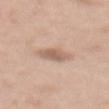Recorded during total-body skin imaging; not selected for excision or biopsy. A 15 mm close-up extracted from a 3D total-body photography capture. The lesion is located on the abdomen. The total-body-photography lesion software estimated an outline eccentricity of about 0.65 (0 = round, 1 = elongated) and a shape-asymmetry score of about 0.2 (0 = symmetric). The analysis additionally found an average lesion color of about L≈62 a*≈18 b*≈28 (CIELAB), about 10 CIELAB-L* units darker than the surrounding skin, and a normalized border contrast of about 6.5. It also reported a border-irregularity index near 2/10 and peripheral color asymmetry of about 1. A female subject aged 38 to 42.